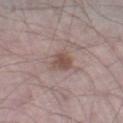Clinical impression: No biopsy was performed on this lesion — it was imaged during a full skin examination and was not determined to be concerning. Background: The lesion's longest dimension is about 3.5 mm. An algorithmic analysis of the crop reported a footprint of about 5.5 mm² and two-axis asymmetry of about 0.25. The analysis additionally found border irregularity of about 2.5 on a 0–10 scale, a color-variation rating of about 3/10, and peripheral color asymmetry of about 1. Cropped from a total-body skin-imaging series; the visible field is about 15 mm. A male patient in their 70s. On the right lower leg.